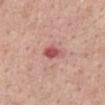This lesion was catalogued during total-body skin photography and was not selected for biopsy. A region of skin cropped from a whole-body photographic capture, roughly 15 mm wide. The subject is a male in their mid- to late 50s. The lesion is on the chest. This is a white-light tile. Automated tile analysis of the lesion measured a lesion area of about 4 mm², an outline eccentricity of about 0.7 (0 = round, 1 = elongated), and a symmetry-axis asymmetry near 0.25. The software also gave a lesion color around L≈54 a*≈31 b*≈24 in CIELAB and roughly 13 lightness units darker than nearby skin.